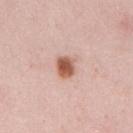| field | value |
|---|---|
| biopsy status | catalogued during a skin exam; not biopsied |
| diameter | about 2.5 mm |
| site | the upper back |
| patient | male, roughly 25 years of age |
| illumination | white-light |
| acquisition | 15 mm crop, total-body photography |
| automated metrics | a border-irregularity index near 2/10 and a color-variation rating of about 4.5/10; a classifier nevus-likeness of about 100/100 and a lesion-detection confidence of about 100/100 |